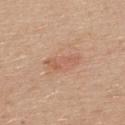Background: The subject is a female about 30 years old. A region of skin cropped from a whole-body photographic capture, roughly 15 mm wide. Located on the upper back. Imaged with white-light lighting.